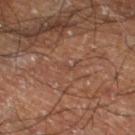{
  "patient": {
    "sex": "male",
    "age_approx": 60
  },
  "automated_metrics": {
    "area_mm2_approx": 1.0,
    "border_irregularity_0_10": 4.0,
    "peripheral_color_asymmetry": 0.0,
    "nevus_likeness_0_100": 0
  },
  "lighting": "cross-polarized",
  "lesion_size": {
    "long_diameter_mm_approx": 1.5
  },
  "site": "left lower leg",
  "image": {
    "source": "total-body photography crop",
    "field_of_view_mm": 15
  }
}